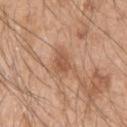No biopsy was performed on this lesion — it was imaged during a full skin examination and was not determined to be concerning. The lesion is on the left upper arm. Cropped from a total-body skin-imaging series; the visible field is about 15 mm. The tile uses white-light illumination. About 3.5 mm across. An algorithmic analysis of the crop reported a border-irregularity rating of about 5.5/10 and peripheral color asymmetry of about 0.5. The patient is a male about 50 years old.